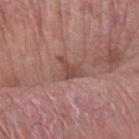Clinical impression: Recorded during total-body skin imaging; not selected for excision or biopsy. Acquisition and patient details: This image is a 15 mm lesion crop taken from a total-body photograph. The lesion is on the left forearm. A male patient about 75 years old.